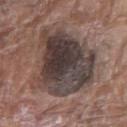Q: Was a biopsy performed?
A: total-body-photography surveillance lesion; no biopsy
Q: Patient demographics?
A: male, about 80 years old
Q: How large is the lesion?
A: ≈9 mm
Q: How was the tile lit?
A: white-light illumination
Q: What kind of image is this?
A: ~15 mm tile from a whole-body skin photo
Q: Lesion location?
A: the chest